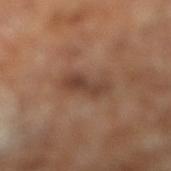<tbp_lesion>
<biopsy_status>not biopsied; imaged during a skin examination</biopsy_status>
<image>
  <source>total-body photography crop</source>
  <field_of_view_mm>15</field_of_view_mm>
</image>
<automated_metrics>
  <border_irregularity_0_10>3.0</border_irregularity_0_10>
  <color_variation_0_10>3.0</color_variation_0_10>
  <peripheral_color_asymmetry>1.0</peripheral_color_asymmetry>
</automated_metrics>
<lighting>cross-polarized</lighting>
<patient>
  <sex>male</sex>
  <age_approx>65</age_approx>
</patient>
</tbp_lesion>